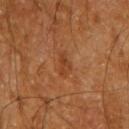<record>
<biopsy_status>not biopsied; imaged during a skin examination</biopsy_status>
<site>right upper arm</site>
<lesion_size>
  <long_diameter_mm_approx>2.5</long_diameter_mm_approx>
</lesion_size>
<patient>
  <sex>male</sex>
  <age_approx>60</age_approx>
</patient>
<image>
  <source>total-body photography crop</source>
  <field_of_view_mm>15</field_of_view_mm>
</image>
</record>